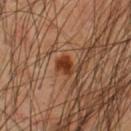Part of a total-body skin-imaging series; this lesion was reviewed on a skin check and was not flagged for biopsy. The subject is a male aged approximately 55. On the chest. A roughly 15 mm field-of-view crop from a total-body skin photograph.